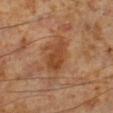follow-up = catalogued during a skin exam; not biopsied
lesion size = about 4.5 mm
imaging modality = ~15 mm crop, total-body skin-cancer survey
location = the left lower leg
illumination = cross-polarized
subject = male, aged approximately 60